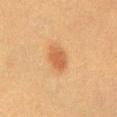Clinical impression: Part of a total-body skin-imaging series; this lesion was reviewed on a skin check and was not flagged for biopsy. Background: A lesion tile, about 15 mm wide, cut from a 3D total-body photograph. Longest diameter approximately 3 mm. The total-body-photography lesion software estimated border irregularity of about 1.5 on a 0–10 scale and a within-lesion color-variation index near 2.5/10. The software also gave a classifier nevus-likeness of about 100/100 and a lesion-detection confidence of about 100/100. This is a cross-polarized tile. The subject is a female aged 53–57. Located on the abdomen.